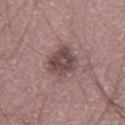{"biopsy_status": "not biopsied; imaged during a skin examination", "image": {"source": "total-body photography crop", "field_of_view_mm": 15}, "lighting": "white-light", "lesion_size": {"long_diameter_mm_approx": 4.0}, "site": "right thigh", "patient": {"sex": "male", "age_approx": 60}}